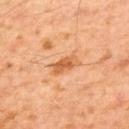No biopsy was performed on this lesion — it was imaged during a full skin examination and was not determined to be concerning. A male subject aged 58–62. About 3 mm across. The lesion is on the upper back. Imaged with cross-polarized lighting. The total-body-photography lesion software estimated a mean CIELAB color near L≈57 a*≈26 b*≈40 and a lesion–skin lightness drop of about 10. It also reported a border-irregularity index near 2.5/10, a within-lesion color-variation index near 3/10, and peripheral color asymmetry of about 1. The analysis additionally found a classifier nevus-likeness of about 5/100 and a lesion-detection confidence of about 100/100. A region of skin cropped from a whole-body photographic capture, roughly 15 mm wide.